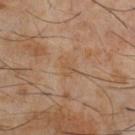biopsy_status: not biopsied; imaged during a skin examination
automated_metrics:
  vs_skin_contrast_norm: 4.5
  border_irregularity_0_10: 3.0
  color_variation_0_10: 1.0
  peripheral_color_asymmetry: 0.5
patient:
  sex: male
  age_approx: 60
image:
  source: total-body photography crop
  field_of_view_mm: 15
site: chest
lesion_size:
  long_diameter_mm_approx: 2.5
lighting: cross-polarized